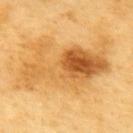The lesion was tiled from a total-body skin photograph and was not biopsied. A roughly 15 mm field-of-view crop from a total-body skin photograph. A male patient, approximately 60 years of age. Located on the upper back. Imaged with cross-polarized lighting.